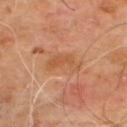{"biopsy_status": "not biopsied; imaged during a skin examination", "lesion_size": {"long_diameter_mm_approx": 4.5}, "site": "upper back", "lighting": "cross-polarized", "image": {"source": "total-body photography crop", "field_of_view_mm": 15}, "automated_metrics": {"border_irregularity_0_10": 4.0, "color_variation_0_10": 4.5, "nevus_likeness_0_100": 0, "lesion_detection_confidence_0_100": 100}, "patient": {"sex": "male", "age_approx": 65}}